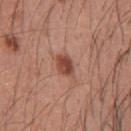Notes:
* workup — no biopsy performed (imaged during a skin exam)
* size — ~3 mm (longest diameter)
* illumination — white-light
* site — the upper back
* patient — male, aged approximately 45
* image — ~15 mm tile from a whole-body skin photo